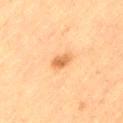Part of a total-body skin-imaging series; this lesion was reviewed on a skin check and was not flagged for biopsy.
The total-body-photography lesion software estimated a mean CIELAB color near L≈54 a*≈21 b*≈38, a lesion–skin lightness drop of about 10, and a lesion-to-skin contrast of about 7.5 (normalized; higher = more distinct). The software also gave a border-irregularity index near 2.5/10 and peripheral color asymmetry of about 1. The analysis additionally found a nevus-likeness score of about 85/100.
From the left thigh.
A lesion tile, about 15 mm wide, cut from a 3D total-body photograph.
The subject is a male aged 73–77.